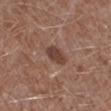Recorded during total-body skin imaging; not selected for excision or biopsy.
The tile uses white-light illumination.
An algorithmic analysis of the crop reported a lesion area of about 4.5 mm², an eccentricity of roughly 0.8, and a symmetry-axis asymmetry near 0.25. The software also gave a border-irregularity rating of about 2/10, a color-variation rating of about 2.5/10, and radial color variation of about 1.
The lesion's longest dimension is about 3 mm.
The subject is a male aged 43 to 47.
A region of skin cropped from a whole-body photographic capture, roughly 15 mm wide.
The lesion is located on the right lower leg.